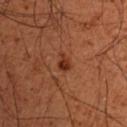follow-up=imaged on a skin check; not biopsied
anatomic site=the chest
subject=male, aged around 50
acquisition=total-body-photography crop, ~15 mm field of view
tile lighting=cross-polarized illumination